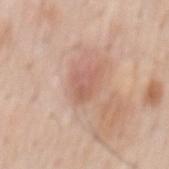{"biopsy_status": "not biopsied; imaged during a skin examination", "lesion_size": {"long_diameter_mm_approx": 3.5}, "image": {"source": "total-body photography crop", "field_of_view_mm": 15}, "lighting": "white-light", "patient": {"sex": "male", "age_approx": 60}, "site": "mid back"}